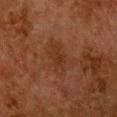Q: Was a biopsy performed?
A: imaged on a skin check; not biopsied
Q: What are the patient's age and sex?
A: female, aged 48–52
Q: Lesion location?
A: the chest
Q: How was this image acquired?
A: ~15 mm crop, total-body skin-cancer survey